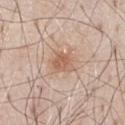Clinical impression:
The lesion was photographed on a routine skin check and not biopsied; there is no pathology result.
Clinical summary:
The lesion is on the front of the torso. A male subject, in their 80s. This is a white-light tile. A region of skin cropped from a whole-body photographic capture, roughly 15 mm wide. Measured at roughly 2.5 mm in maximum diameter.